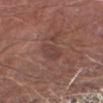Assessment:
The lesion was photographed on a routine skin check and not biopsied; there is no pathology result.